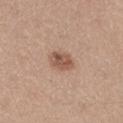<case>
<biopsy_status>not biopsied; imaged during a skin examination</biopsy_status>
<lesion_size>
  <long_diameter_mm_approx>3.5</long_diameter_mm_approx>
</lesion_size>
<site>left lower leg</site>
<image>
  <source>total-body photography crop</source>
  <field_of_view_mm>15</field_of_view_mm>
</image>
<lighting>white-light</lighting>
<patient>
  <sex>female</sex>
  <age_approx>45</age_approx>
</patient>
</case>